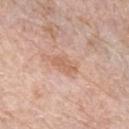Q: Was a biopsy performed?
A: catalogued during a skin exam; not biopsied
Q: Who is the patient?
A: female, aged 63–67
Q: What kind of image is this?
A: ~15 mm tile from a whole-body skin photo
Q: How was the tile lit?
A: white-light illumination
Q: Automated lesion metrics?
A: a lesion area of about 4.5 mm², an outline eccentricity of about 0.9 (0 = round, 1 = elongated), and two-axis asymmetry of about 0.4; a lesion–skin lightness drop of about 8 and a normalized lesion–skin contrast near 6; border irregularity of about 4 on a 0–10 scale, a color-variation rating of about 1/10, and radial color variation of about 0.5
Q: Lesion size?
A: ≈3.5 mm
Q: Where on the body is the lesion?
A: the left forearm